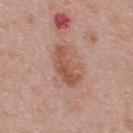From the upper back.
This is a white-light tile.
About 5 mm across.
A 15 mm close-up tile from a total-body photography series done for melanoma screening.
An algorithmic analysis of the crop reported a within-lesion color-variation index near 4.5/10 and peripheral color asymmetry of about 1.5. The analysis additionally found a classifier nevus-likeness of about 15/100.
A male subject, aged around 45.